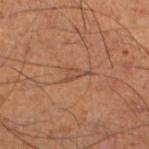Case summary:
– workup: imaged on a skin check; not biopsied
– location: the left lower leg
– lighting: cross-polarized illumination
– lesion diameter: about 3.5 mm
– subject: male, roughly 50 years of age
– acquisition: ~15 mm crop, total-body skin-cancer survey